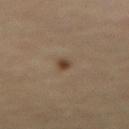Notes:
* follow-up — catalogued during a skin exam; not biopsied
* body site — the abdomen
* imaging modality — ~15 mm tile from a whole-body skin photo
* subject — female, in their mid-60s